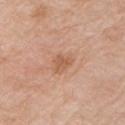This lesion was catalogued during total-body skin photography and was not selected for biopsy.
An algorithmic analysis of the crop reported a lesion area of about 5 mm², a shape eccentricity near 0.65, and a shape-asymmetry score of about 0.35 (0 = symmetric). The software also gave a lesion color around L≈59 a*≈22 b*≈33 in CIELAB. It also reported border irregularity of about 3.5 on a 0–10 scale, a within-lesion color-variation index near 2/10, and a peripheral color-asymmetry measure near 0.5. The software also gave a classifier nevus-likeness of about 0/100 and a lesion-detection confidence of about 100/100.
The subject is a male aged around 80.
A roughly 15 mm field-of-view crop from a total-body skin photograph.
Located on the left upper arm.
The tile uses white-light illumination.